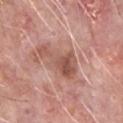Q: Is there a histopathology result?
A: catalogued during a skin exam; not biopsied
Q: What is the imaging modality?
A: ~15 mm tile from a whole-body skin photo
Q: Where on the body is the lesion?
A: the chest
Q: What did automated image analysis measure?
A: an area of roughly 9.5 mm², a shape eccentricity near 0.9, and a shape-asymmetry score of about 0.6 (0 = symmetric); a lesion color around L≈53 a*≈23 b*≈27 in CIELAB and about 10 CIELAB-L* units darker than the surrounding skin; internal color variation of about 4 on a 0–10 scale and peripheral color asymmetry of about 1.5; a nevus-likeness score of about 0/100 and lesion-presence confidence of about 100/100
Q: What lighting was used for the tile?
A: white-light illumination
Q: Lesion size?
A: about 5.5 mm
Q: What are the patient's age and sex?
A: male, roughly 60 years of age